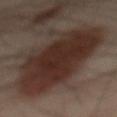notes: total-body-photography surveillance lesion; no biopsy
illumination: cross-polarized
anatomic site: the mid back
acquisition: 15 mm crop, total-body photography
diameter: ≈13 mm
subject: male, aged approximately 50
automated metrics: a mean CIELAB color near L≈29 a*≈15 b*≈20, about 12 CIELAB-L* units darker than the surrounding skin, and a normalized lesion–skin contrast near 11.5; a nevus-likeness score of about 100/100 and a lesion-detection confidence of about 100/100